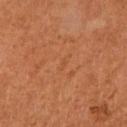workup = imaged on a skin check; not biopsied
image source = total-body-photography crop, ~15 mm field of view
lighting = cross-polarized illumination
subject = female, aged 63–67
anatomic site = the arm
automated metrics = an average lesion color of about L≈48 a*≈26 b*≈38 (CIELAB) and a normalized border contrast of about 3; border irregularity of about 3.5 on a 0–10 scale, a color-variation rating of about 0/10, and peripheral color asymmetry of about 0
size = about 1.5 mm